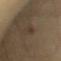Findings:
• workup: no biopsy performed (imaged during a skin exam)
• illumination: cross-polarized
• acquisition: 15 mm crop, total-body photography
• TBP lesion metrics: border irregularity of about 4 on a 0–10 scale, internal color variation of about 0 on a 0–10 scale, and a peripheral color-asymmetry measure near 0; an automated nevus-likeness rating near 5 out of 100 and a lesion-detection confidence of about 85/100
• anatomic site: the chest
• subject: female, in their 60s